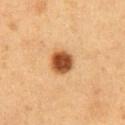Clinical impression: The lesion was tiled from a total-body skin photograph and was not biopsied. Background: Located on the front of the torso. A roughly 15 mm field-of-view crop from a total-body skin photograph. A male patient aged 53–57. The recorded lesion diameter is about 3 mm.